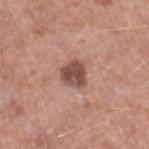Clinical impression:
The lesion was photographed on a routine skin check and not biopsied; there is no pathology result.
Acquisition and patient details:
About 3 mm across. A male subject roughly 50 years of age. A roughly 15 mm field-of-view crop from a total-body skin photograph. This is a white-light tile. The lesion is located on the right lower leg. The total-body-photography lesion software estimated an area of roughly 6.5 mm², a shape eccentricity near 0.3, and a symmetry-axis asymmetry near 0.25. It also reported a border-irregularity index near 2/10, internal color variation of about 3 on a 0–10 scale, and peripheral color asymmetry of about 1.